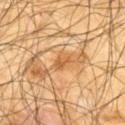follow-up: catalogued during a skin exam; not biopsied
anatomic site: the upper back
patient: male, in their mid- to late 60s
lighting: cross-polarized
diameter: about 2.5 mm
acquisition: 15 mm crop, total-body photography